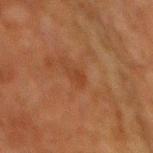Case summary:
- workup: catalogued during a skin exam; not biopsied
- subject: male, aged approximately 80
- automated lesion analysis: a lesion area of about 2.5 mm², an outline eccentricity of about 0.9 (0 = round, 1 = elongated), and two-axis asymmetry of about 0.45; a color-variation rating of about 0.5/10 and radial color variation of about 0
- acquisition: ~15 mm crop, total-body skin-cancer survey
- lesion diameter: about 2.5 mm
- anatomic site: the mid back
- illumination: cross-polarized illumination